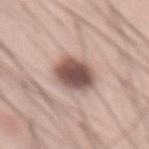Imaged during a routine full-body skin examination; the lesion was not biopsied and no histopathology is available. An algorithmic analysis of the crop reported a lesion color around L≈52 a*≈18 b*≈22 in CIELAB, roughly 18 lightness units darker than nearby skin, and a lesion-to-skin contrast of about 12 (normalized; higher = more distinct). It also reported a border-irregularity index near 1.5/10, a within-lesion color-variation index near 5/10, and peripheral color asymmetry of about 1. Captured under white-light illumination. On the front of the torso. Measured at roughly 4.5 mm in maximum diameter. A 15 mm crop from a total-body photograph taken for skin-cancer surveillance. A male patient, aged approximately 60.